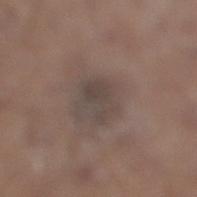<lesion>
<biopsy_status>not biopsied; imaged during a skin examination</biopsy_status>
<site>leg</site>
<patient>
  <sex>male</sex>
  <age_approx>65</age_approx>
</patient>
<image>
  <source>total-body photography crop</source>
  <field_of_view_mm>15</field_of_view_mm>
</image>
<lighting>white-light</lighting>
</lesion>